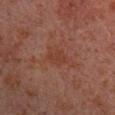notes = total-body-photography surveillance lesion; no biopsy | image source = ~15 mm tile from a whole-body skin photo | location = the right upper arm | subject = male, approximately 30 years of age.